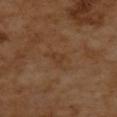Recorded during total-body skin imaging; not selected for excision or biopsy. A 15 mm close-up tile from a total-body photography series done for melanoma screening. The lesion is on the upper back. The lesion-visualizer software estimated a lesion area of about 2.5 mm², an outline eccentricity of about 0.85 (0 = round, 1 = elongated), and a symmetry-axis asymmetry near 0.45. And it measured internal color variation of about 0 on a 0–10 scale. And it measured an automated nevus-likeness rating near 0 out of 100 and a detector confidence of about 100 out of 100 that the crop contains a lesion. A female subject, in their mid- to late 50s. The tile uses cross-polarized illumination. The recorded lesion diameter is about 2.5 mm.